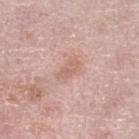{"biopsy_status": "not biopsied; imaged during a skin examination", "patient": {"sex": "female", "age_approx": 60}, "site": "left lower leg", "image": {"source": "total-body photography crop", "field_of_view_mm": 15}}